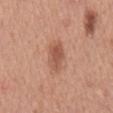* follow-up · no biopsy performed (imaged during a skin exam)
* tile lighting · white-light illumination
* anatomic site · the back
* lesion diameter · ≈4 mm
* image · ~15 mm tile from a whole-body skin photo
* patient · male, roughly 65 years of age